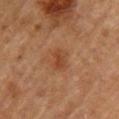The recorded lesion diameter is about 3 mm.
A region of skin cropped from a whole-body photographic capture, roughly 15 mm wide.
A female patient, aged around 60.
Captured under cross-polarized illumination.
On the upper back.